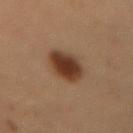Q: Is there a histopathology result?
A: no biopsy performed (imaged during a skin exam)
Q: How was the tile lit?
A: cross-polarized
Q: How was this image acquired?
A: 15 mm crop, total-body photography
Q: Lesion size?
A: ~4.5 mm (longest diameter)
Q: Who is the patient?
A: female
Q: Where on the body is the lesion?
A: the lower back